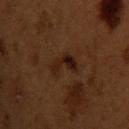Impression:
Recorded during total-body skin imaging; not selected for excision or biopsy.
Image and clinical context:
The lesion-visualizer software estimated a mean CIELAB color near L≈15 a*≈15 b*≈18 and a lesion–skin lightness drop of about 6. The analysis additionally found a nevus-likeness score of about 5/100 and a detector confidence of about 100 out of 100 that the crop contains a lesion. Located on the chest. The subject is a male aged around 50. A lesion tile, about 15 mm wide, cut from a 3D total-body photograph.